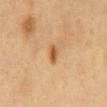{"biopsy_status": "not biopsied; imaged during a skin examination", "patient": {"sex": "male", "age_approx": 60}, "lighting": "cross-polarized", "image": {"source": "total-body photography crop", "field_of_view_mm": 15}, "automated_metrics": {"cielab_L": 57, "cielab_a": 21, "cielab_b": 40, "vs_skin_darker_L": 11.0, "vs_skin_contrast_norm": 8.0, "border_irregularity_0_10": 2.0, "peripheral_color_asymmetry": 2.0, "nevus_likeness_0_100": 90}, "site": "abdomen", "lesion_size": {"long_diameter_mm_approx": 2.5}}